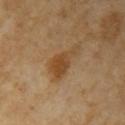Imaged during a routine full-body skin examination; the lesion was not biopsied and no histopathology is available.